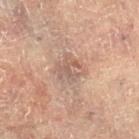No biopsy was performed on this lesion — it was imaged during a full skin examination and was not determined to be concerning. The subject is a female aged 78 to 82. Imaged with cross-polarized lighting. Automated tile analysis of the lesion measured a footprint of about 9 mm², a shape eccentricity near 0.2, and a shape-asymmetry score of about 0.2 (0 = symmetric). It also reported internal color variation of about 4.5 on a 0–10 scale and peripheral color asymmetry of about 1.5. Located on the leg. A roughly 15 mm field-of-view crop from a total-body skin photograph. Approximately 3.5 mm at its widest.